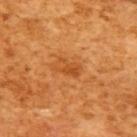The lesion was tiled from a total-body skin photograph and was not biopsied.
A region of skin cropped from a whole-body photographic capture, roughly 15 mm wide.
Located on the right upper arm.
The tile uses cross-polarized illumination.
A female patient, roughly 55 years of age.
About 3 mm across.